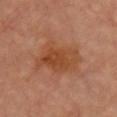| feature | finding |
|---|---|
| workup | no biopsy performed (imaged during a skin exam) |
| location | the chest |
| imaging modality | ~15 mm crop, total-body skin-cancer survey |
| lesion size | about 5.5 mm |
| patient | male, roughly 65 years of age |
| image-analysis metrics | a mean CIELAB color near L≈46 a*≈24 b*≈35 and a normalized border contrast of about 7; an automated nevus-likeness rating near 30 out of 100 and a detector confidence of about 100 out of 100 that the crop contains a lesion |